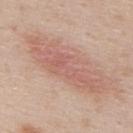follow-up = no biopsy performed (imaged during a skin exam); diameter = about 10 mm; illumination = white-light illumination; image source = ~15 mm tile from a whole-body skin photo; subject = male, aged 38–42; location = the upper back.